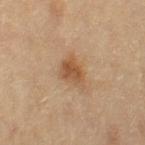Captured during whole-body skin photography for melanoma surveillance; the lesion was not biopsied.
The lesion's longest dimension is about 4 mm.
From the right thigh.
Imaged with cross-polarized lighting.
This image is a 15 mm lesion crop taken from a total-body photograph.
The subject is a female aged 78 to 82.